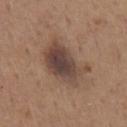Impression: Recorded during total-body skin imaging; not selected for excision or biopsy. Acquisition and patient details: The lesion is located on the chest. The lesion's longest dimension is about 6 mm. A roughly 15 mm field-of-view crop from a total-body skin photograph. Imaged with white-light lighting. A male subject about 65 years old.